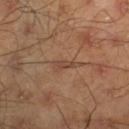Notes:
– biopsy status — catalogued during a skin exam; not biopsied
– patient — male, aged 43–47
– body site — the leg
– imaging modality — ~15 mm crop, total-body skin-cancer survey